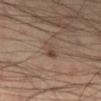* biopsy status: imaged on a skin check; not biopsied
* body site: the right lower leg
* diameter: about 2.5 mm
* subject: male, in their mid- to late 30s
* image: ~15 mm crop, total-body skin-cancer survey